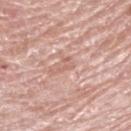The lesion was tiled from a total-body skin photograph and was not biopsied.
The total-body-photography lesion software estimated a lesion–skin lightness drop of about 7. The software also gave a border-irregularity rating of about 7/10, a color-variation rating of about 0/10, and a peripheral color-asymmetry measure near 0. The analysis additionally found a detector confidence of about 70 out of 100 that the crop contains a lesion.
The tile uses white-light illumination.
A 15 mm close-up tile from a total-body photography series done for melanoma screening.
The lesion's longest dimension is about 2.5 mm.
From the right upper arm.
A female patient, aged around 60.